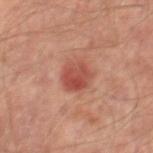The lesion was tiled from a total-body skin photograph and was not biopsied.
Automated tile analysis of the lesion measured lesion-presence confidence of about 100/100.
Captured under cross-polarized illumination.
The lesion is located on the right thigh.
The patient is a male aged 63–67.
Approximately 3 mm at its widest.
A 15 mm close-up extracted from a 3D total-body photography capture.